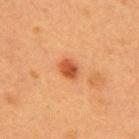<case>
  <biopsy_status>not biopsied; imaged during a skin examination</biopsy_status>
  <lighting>cross-polarized</lighting>
  <site>upper back</site>
  <patient>
    <sex>female</sex>
    <age_approx>40</age_approx>
  </patient>
  <automated_metrics>
    <area_mm2_approx>4.5</area_mm2_approx>
    <eccentricity>0.65</eccentricity>
    <shape_asymmetry>0.1</shape_asymmetry>
    <border_irregularity_0_10>1.0</border_irregularity_0_10>
    <color_variation_0_10>3.0</color_variation_0_10>
    <peripheral_color_asymmetry>1.0</peripheral_color_asymmetry>
  </automated_metrics>
  <lesion_size>
    <long_diameter_mm_approx>2.5</long_diameter_mm_approx>
  </lesion_size>
  <image>
    <source>total-body photography crop</source>
    <field_of_view_mm>15</field_of_view_mm>
  </image>
</case>